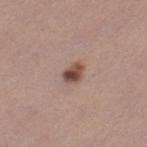The lesion was photographed on a routine skin check and not biopsied; there is no pathology result. A female subject, in their 30s. A close-up tile cropped from a whole-body skin photograph, about 15 mm across. The lesion is located on the leg. Longest diameter approximately 2.5 mm.